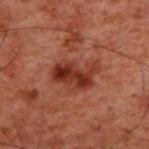Clinical impression: Captured during whole-body skin photography for melanoma surveillance; the lesion was not biopsied. Image and clinical context: On the upper back. Cropped from a whole-body photographic skin survey; the tile spans about 15 mm. This is a cross-polarized tile. The subject is a male aged around 60.